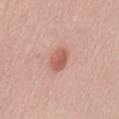Clinical summary: Measured at roughly 3 mm in maximum diameter. A 15 mm crop from a total-body photograph taken for skin-cancer surveillance. Automated tile analysis of the lesion measured a lesion area of about 5.5 mm², an outline eccentricity of about 0.6 (0 = round, 1 = elongated), and a shape-asymmetry score of about 0.15 (0 = symmetric). The analysis additionally found a color-variation rating of about 3/10 and radial color variation of about 1. Imaged with white-light lighting. The lesion is located on the lower back. A female subject aged 43–47.